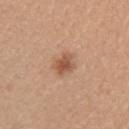{"site": "right upper arm", "image": {"source": "total-body photography crop", "field_of_view_mm": 15}, "patient": {"sex": "female", "age_approx": 30}}